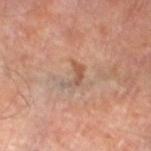Part of a total-body skin-imaging series; this lesion was reviewed on a skin check and was not flagged for biopsy. Automated image analysis of the tile measured an average lesion color of about L≈53 a*≈19 b*≈29 (CIELAB), roughly 8 lightness units darker than nearby skin, and a normalized lesion–skin contrast near 6. It also reported border irregularity of about 7.5 on a 0–10 scale, a color-variation rating of about 0/10, and radial color variation of about 0. It also reported a nevus-likeness score of about 0/100 and lesion-presence confidence of about 95/100. The tile uses cross-polarized illumination. A lesion tile, about 15 mm wide, cut from a 3D total-body photograph. The lesion is on the left lower leg. A male patient, in their 70s.